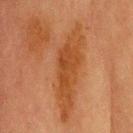{"biopsy_status": "not biopsied; imaged during a skin examination", "site": "head or neck", "image": {"source": "total-body photography crop", "field_of_view_mm": 15}, "patient": {"sex": "male", "age_approx": 70}}